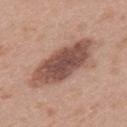follow-up: no biopsy performed (imaged during a skin exam) | location: the upper back | image-analysis metrics: an area of roughly 25 mm², an eccentricity of roughly 0.9, and a symmetry-axis asymmetry near 0.15; an average lesion color of about L≈50 a*≈20 b*≈25 (CIELAB), roughly 15 lightness units darker than nearby skin, and a lesion-to-skin contrast of about 10 (normalized; higher = more distinct); a border-irregularity index near 2.5/10, a within-lesion color-variation index near 4.5/10, and radial color variation of about 1.5 | image: 15 mm crop, total-body photography | illumination: white-light illumination | diameter: ≈8.5 mm | subject: female, aged approximately 40.